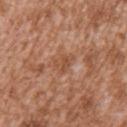biopsy status: imaged on a skin check; not biopsied
anatomic site: the left upper arm
subject: male, in their mid-40s
tile lighting: white-light illumination
imaging modality: ~15 mm tile from a whole-body skin photo
diameter: about 3 mm